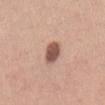biopsy status: catalogued during a skin exam; not biopsied | subject: male, aged 28 to 32 | tile lighting: white-light illumination | diameter: ~3.5 mm (longest diameter) | automated metrics: a mean CIELAB color near L≈53 a*≈21 b*≈25, about 16 CIELAB-L* units darker than the surrounding skin, and a lesion-to-skin contrast of about 10.5 (normalized; higher = more distinct); a border-irregularity index near 1.5/10, a color-variation rating of about 2.5/10, and radial color variation of about 0.5; a lesion-detection confidence of about 100/100 | image source: 15 mm crop, total-body photography | location: the back.